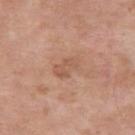<case>
  <biopsy_status>not biopsied; imaged during a skin examination</biopsy_status>
  <lighting>white-light</lighting>
  <image>
    <source>total-body photography crop</source>
    <field_of_view_mm>15</field_of_view_mm>
  </image>
  <patient>
    <sex>male</sex>
    <age_approx>55</age_approx>
  </patient>
  <site>right upper arm</site>
  <lesion_size>
    <long_diameter_mm_approx>3.5</long_diameter_mm_approx>
  </lesion_size>
</case>